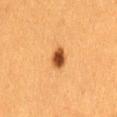<record>
  <biopsy_status>not biopsied; imaged during a skin examination</biopsy_status>
  <patient>
    <sex>female</sex>
    <age_approx>40</age_approx>
  </patient>
  <image>
    <source>total-body photography crop</source>
    <field_of_view_mm>15</field_of_view_mm>
  </image>
  <lighting>cross-polarized</lighting>
  <site>lower back</site>
</record>